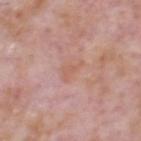The lesion was photographed on a routine skin check and not biopsied; there is no pathology result.
Captured under white-light illumination.
From the head or neck.
Cropped from a total-body skin-imaging series; the visible field is about 15 mm.
A male patient, aged 68 to 72.